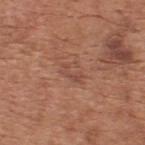  biopsy_status: not biopsied; imaged during a skin examination